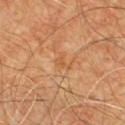workup = imaged on a skin check; not biopsied | size = ~2.5 mm (longest diameter) | image source = total-body-photography crop, ~15 mm field of view | subject = male, about 60 years old | anatomic site = the chest | lighting = cross-polarized illumination.